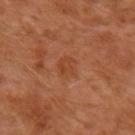This lesion was catalogued during total-body skin photography and was not selected for biopsy.
Cropped from a total-body skin-imaging series; the visible field is about 15 mm.
Longest diameter approximately 2.5 mm.
On the left forearm.
The patient is a male aged around 30.
The tile uses cross-polarized illumination.
An algorithmic analysis of the crop reported a footprint of about 5 mm² and a shape eccentricity near 0.55. And it measured a lesion color around L≈43 a*≈25 b*≈35 in CIELAB, a lesion–skin lightness drop of about 6, and a normalized lesion–skin contrast near 5. The analysis additionally found a color-variation rating of about 2.5/10 and peripheral color asymmetry of about 1. It also reported a classifier nevus-likeness of about 0/100 and a lesion-detection confidence of about 100/100.